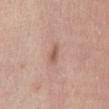Recorded during total-body skin imaging; not selected for excision or biopsy.